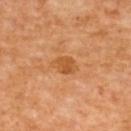Q: Was a biopsy performed?
A: imaged on a skin check; not biopsied
Q: Who is the patient?
A: female, aged approximately 50
Q: Lesion location?
A: the upper back
Q: What is the imaging modality?
A: total-body-photography crop, ~15 mm field of view
Q: What lighting was used for the tile?
A: cross-polarized illumination
Q: What is the lesion's diameter?
A: ~3 mm (longest diameter)
Q: Automated lesion metrics?
A: a footprint of about 5 mm², a shape eccentricity near 0.75, and a symmetry-axis asymmetry near 0.25